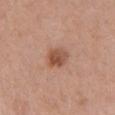biopsy status=imaged on a skin check; not biopsied
lesion size=≈3.5 mm
site=the chest
image source=total-body-photography crop, ~15 mm field of view
tile lighting=white-light
patient=male, in their 70s